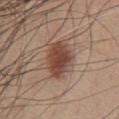follow-up — total-body-photography surveillance lesion; no biopsy | imaging modality — ~15 mm tile from a whole-body skin photo | lesion diameter — about 5 mm | location — the front of the torso | patient — male, approximately 55 years of age | tile lighting — white-light illumination.